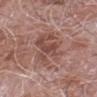Findings:
* biopsy status · imaged on a skin check; not biopsied
* tile lighting · white-light
* patient · male, in their mid-70s
* image source · 15 mm crop, total-body photography
* location · the arm